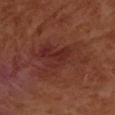This is a cross-polarized tile. The patient is a female aged 53 to 57. An algorithmic analysis of the crop reported a lesion area of about 22 mm², a shape eccentricity near 0.85, and two-axis asymmetry of about 0.5. The software also gave a nevus-likeness score of about 0/100. This image is a 15 mm lesion crop taken from a total-body photograph. From the left forearm. The lesion's longest dimension is about 7.5 mm.